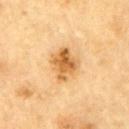workup: imaged on a skin check; not biopsied | automated metrics: a lesion area of about 10 mm², a shape eccentricity near 0.6, and two-axis asymmetry of about 0.2; an average lesion color of about L≈55 a*≈19 b*≈41 (CIELAB) and a normalized border contrast of about 8.5; a border-irregularity rating of about 2/10 and peripheral color asymmetry of about 3; an automated nevus-likeness rating near 85 out of 100 and a detector confidence of about 100 out of 100 that the crop contains a lesion | image: 15 mm crop, total-body photography | size: about 4 mm | illumination: cross-polarized | anatomic site: the left upper arm | subject: male, aged approximately 85 or older.